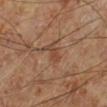Impression: No biopsy was performed on this lesion — it was imaged during a full skin examination and was not determined to be concerning. Acquisition and patient details: Cropped from a total-body skin-imaging series; the visible field is about 15 mm. The patient is a male aged 68–72. The total-body-photography lesion software estimated a lesion area of about 3 mm², a shape eccentricity near 0.85, and two-axis asymmetry of about 0.35. And it measured a lesion color around L≈43 a*≈20 b*≈29 in CIELAB, a lesion–skin lightness drop of about 7, and a normalized border contrast of about 6. It also reported a border-irregularity rating of about 4/10, a within-lesion color-variation index near 0.5/10, and a peripheral color-asymmetry measure near 0. The analysis additionally found an automated nevus-likeness rating near 15 out of 100 and a lesion-detection confidence of about 100/100. The lesion's longest dimension is about 2.5 mm. From the leg.